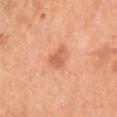Assessment:
The lesion was tiled from a total-body skin photograph and was not biopsied.
Image and clinical context:
Longest diameter approximately 2.5 mm. The lesion-visualizer software estimated a lesion area of about 4 mm² and a symmetry-axis asymmetry near 0.4. The software also gave a lesion color around L≈62 a*≈28 b*≈37 in CIELAB, roughly 10 lightness units darker than nearby skin, and a normalized border contrast of about 6.5. It also reported a border-irregularity index near 3.5/10 and a peripheral color-asymmetry measure near 1. And it measured an automated nevus-likeness rating near 50 out of 100 and a lesion-detection confidence of about 100/100. The lesion is located on the front of the torso. A male subject aged approximately 50. A 15 mm crop from a total-body photograph taken for skin-cancer surveillance.